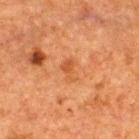No biopsy was performed on this lesion — it was imaged during a full skin examination and was not determined to be concerning. The total-body-photography lesion software estimated an average lesion color of about L≈44 a*≈24 b*≈36 (CIELAB), about 6 CIELAB-L* units darker than the surrounding skin, and a lesion-to-skin contrast of about 5.5 (normalized; higher = more distinct). The software also gave border irregularity of about 4 on a 0–10 scale and a peripheral color-asymmetry measure near 0. The software also gave a classifier nevus-likeness of about 0/100 and a detector confidence of about 100 out of 100 that the crop contains a lesion. Cropped from a total-body skin-imaging series; the visible field is about 15 mm. A male patient aged around 50. The lesion is on the upper back. Measured at roughly 3 mm in maximum diameter. This is a cross-polarized tile.